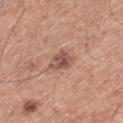The lesion was tiled from a total-body skin photograph and was not biopsied. The lesion is on the left thigh. The tile uses white-light illumination. A 15 mm close-up extracted from a 3D total-body photography capture. Measured at roughly 3 mm in maximum diameter. The patient is a male aged approximately 65.